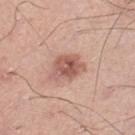Impression: The lesion was tiled from a total-body skin photograph and was not biopsied. Image and clinical context: The lesion is located on the left lower leg. A close-up tile cropped from a whole-body skin photograph, about 15 mm across. A male subject, aged around 40.